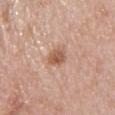automated_metrics:
  area_mm2_approx: 5.0
  shape_asymmetry: 0.25
  nevus_likeness_0_100: 85
  lesion_detection_confidence_0_100: 100
patient:
  sex: female
  age_approx: 45
lesion_size:
  long_diameter_mm_approx: 2.5
lighting: white-light
site: upper back
image:
  source: total-body photography crop
  field_of_view_mm: 15